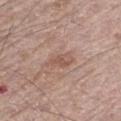Q: Was a biopsy performed?
A: imaged on a skin check; not biopsied
Q: What is the anatomic site?
A: the left thigh
Q: What is the imaging modality?
A: 15 mm crop, total-body photography
Q: What is the lesion's diameter?
A: ~3.5 mm (longest diameter)
Q: Who is the patient?
A: male, roughly 70 years of age
Q: How was the tile lit?
A: white-light illumination